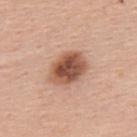No biopsy was performed on this lesion — it was imaged during a full skin examination and was not determined to be concerning.
A 15 mm close-up extracted from a 3D total-body photography capture.
A male patient aged around 55.
Measured at roughly 5 mm in maximum diameter.
The lesion is on the upper back.